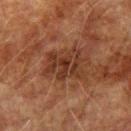{
  "biopsy_status": "not biopsied; imaged during a skin examination",
  "image": {
    "source": "total-body photography crop",
    "field_of_view_mm": 15
  },
  "site": "left upper arm",
  "patient": {
    "sex": "male",
    "age_approx": 75
  },
  "automated_metrics": {
    "area_mm2_approx": 12.0,
    "eccentricity": 0.65,
    "shape_asymmetry": 0.3,
    "nevus_likeness_0_100": 30,
    "lesion_detection_confidence_0_100": 100
  },
  "lesion_size": {
    "long_diameter_mm_approx": 4.5
  },
  "lighting": "cross-polarized"
}